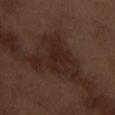biopsy status: catalogued during a skin exam; not biopsied
patient: male, aged approximately 70
imaging modality: total-body-photography crop, ~15 mm field of view
lesion diameter: ~4.5 mm (longest diameter)
anatomic site: the arm
image-analysis metrics: an area of roughly 8 mm², an outline eccentricity of about 0.9 (0 = round, 1 = elongated), and two-axis asymmetry of about 0.3; a mean CIELAB color near L≈22 a*≈18 b*≈20, a lesion–skin lightness drop of about 6, and a lesion-to-skin contrast of about 7 (normalized; higher = more distinct)